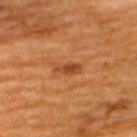image source = ~15 mm crop, total-body skin-cancer survey; patient = male, approximately 60 years of age; site = the upper back; tile lighting = cross-polarized.